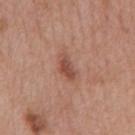Case summary:
– diameter · ~3.5 mm (longest diameter)
– subject · male, in their 70s
– image · total-body-photography crop, ~15 mm field of view
– tile lighting · white-light
– location · the mid back